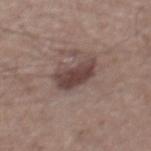notes: total-body-photography surveillance lesion; no biopsy
anatomic site: the abdomen
illumination: white-light illumination
subject: male, aged 53–57
image source: ~15 mm tile from a whole-body skin photo